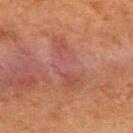No biopsy was performed on this lesion — it was imaged during a full skin examination and was not determined to be concerning.
The tile uses cross-polarized illumination.
A female patient about 50 years old.
Cropped from a whole-body photographic skin survey; the tile spans about 15 mm.
The lesion is located on the back.
The recorded lesion diameter is about 7 mm.